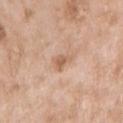biopsy_status: not biopsied; imaged during a skin examination
automated_metrics:
  eccentricity: 0.75
  shape_asymmetry: 0.4
  border_irregularity_0_10: 4.0
  peripheral_color_asymmetry: 1.0
patient:
  sex: female
  age_approx: 75
site: right upper arm
lesion_size:
  long_diameter_mm_approx: 3.0
lighting: white-light
image:
  source: total-body photography crop
  field_of_view_mm: 15